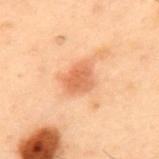Imaged with cross-polarized lighting. Longest diameter approximately 3 mm. A male patient aged approximately 55. Located on the mid back. A roughly 15 mm field-of-view crop from a total-body skin photograph.